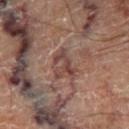biopsy status: total-body-photography surveillance lesion; no biopsy
patient: male, aged approximately 70
illumination: cross-polarized illumination
site: the right thigh
acquisition: ~15 mm tile from a whole-body skin photo